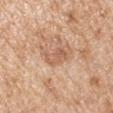biopsy status = total-body-photography surveillance lesion; no biopsy | image-analysis metrics = an automated nevus-likeness rating near 0 out of 100 and a lesion-detection confidence of about 100/100 | lesion diameter = ≈3 mm | site = the arm | lighting = white-light | subject = male, in their mid- to late 60s | imaging modality = ~15 mm tile from a whole-body skin photo.